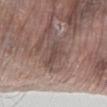Q: Is there a histopathology result?
A: catalogued during a skin exam; not biopsied
Q: What is the imaging modality?
A: 15 mm crop, total-body photography
Q: Patient demographics?
A: female, about 85 years old or older
Q: Lesion location?
A: the left lower leg
Q: Illumination type?
A: white-light illumination
Q: What did automated image analysis measure?
A: a footprint of about 4 mm² and two-axis asymmetry of about 0.45; an average lesion color of about L≈45 a*≈16 b*≈21 (CIELAB), about 8 CIELAB-L* units darker than the surrounding skin, and a normalized lesion–skin contrast near 6; a within-lesion color-variation index near 1/10 and peripheral color asymmetry of about 0.5; a classifier nevus-likeness of about 0/100